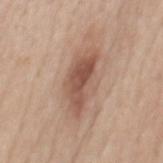Impression: The lesion was tiled from a total-body skin photograph and was not biopsied. Clinical summary: The subject is a male aged approximately 65. From the mid back. A lesion tile, about 15 mm wide, cut from a 3D total-body photograph. Captured under white-light illumination.